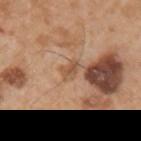Impression: Imaged during a routine full-body skin examination; the lesion was not biopsied and no histopathology is available.